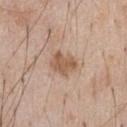Case summary:
• biopsy status: catalogued during a skin exam; not biopsied
• patient: male, aged 58–62
• image-analysis metrics: an area of roughly 6.5 mm², an outline eccentricity of about 0.6 (0 = round, 1 = elongated), and two-axis asymmetry of about 0.25; a classifier nevus-likeness of about 15/100 and a detector confidence of about 100 out of 100 that the crop contains a lesion
• body site: the chest
• acquisition: ~15 mm crop, total-body skin-cancer survey